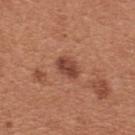The lesion was photographed on a routine skin check and not biopsied; there is no pathology result. A 15 mm crop from a total-body photograph taken for skin-cancer surveillance. Automated image analysis of the tile measured a nevus-likeness score of about 85/100 and a lesion-detection confidence of about 100/100. A female subject aged around 35. The lesion is on the front of the torso. Approximately 3 mm at its widest.